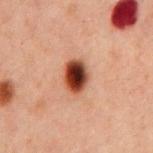Q: What is the anatomic site?
A: the chest
Q: What kind of image is this?
A: total-body-photography crop, ~15 mm field of view
Q: Automated lesion metrics?
A: border irregularity of about 1 on a 0–10 scale and a color-variation rating of about 9/10
Q: Patient demographics?
A: male, aged 58 to 62
Q: Lesion size?
A: about 4 mm